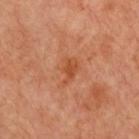Q: Was a biopsy performed?
A: imaged on a skin check; not biopsied
Q: What did automated image analysis measure?
A: a lesion color around L≈42 a*≈24 b*≈32 in CIELAB and a normalized lesion–skin contrast near 6; border irregularity of about 4.5 on a 0–10 scale and radial color variation of about 0.5; an automated nevus-likeness rating near 0 out of 100 and a lesion-detection confidence of about 100/100
Q: What kind of image is this?
A: ~15 mm crop, total-body skin-cancer survey
Q: How large is the lesion?
A: about 3.5 mm
Q: Who is the patient?
A: female, aged approximately 55
Q: What is the anatomic site?
A: the front of the torso